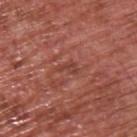This lesion was catalogued during total-body skin photography and was not selected for biopsy. The lesion is located on the back. The patient is a male roughly 65 years of age. A roughly 15 mm field-of-view crop from a total-body skin photograph.